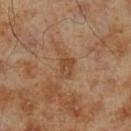Assessment:
No biopsy was performed on this lesion — it was imaged during a full skin examination and was not determined to be concerning.
Image and clinical context:
The recorded lesion diameter is about 3.5 mm. A male patient, aged approximately 70. Imaged with cross-polarized lighting. The lesion is on the leg. The total-body-photography lesion software estimated a shape-asymmetry score of about 0.45 (0 = symmetric). The analysis additionally found a classifier nevus-likeness of about 0/100 and lesion-presence confidence of about 100/100. This image is a 15 mm lesion crop taken from a total-body photograph.